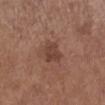Q: Was a biopsy performed?
A: total-body-photography surveillance lesion; no biopsy
Q: What are the patient's age and sex?
A: male, in their mid-70s
Q: Where on the body is the lesion?
A: the left lower leg
Q: What kind of image is this?
A: ~15 mm crop, total-body skin-cancer survey